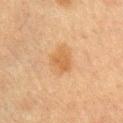Assessment:
No biopsy was performed on this lesion — it was imaged during a full skin examination and was not determined to be concerning.
Image and clinical context:
This is a cross-polarized tile. Longest diameter approximately 2.5 mm. A 15 mm close-up tile from a total-body photography series done for melanoma screening. Automated image analysis of the tile measured a border-irregularity rating of about 3/10, a within-lesion color-variation index near 1.5/10, and radial color variation of about 0.5. The analysis additionally found a lesion-detection confidence of about 100/100. The lesion is located on the left upper arm. The subject is a male aged 63 to 67.